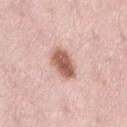Measured at roughly 4 mm in maximum diameter. Imaged with white-light lighting. Automated image analysis of the tile measured a footprint of about 8.5 mm², an outline eccentricity of about 0.75 (0 = round, 1 = elongated), and two-axis asymmetry of about 0.2. And it measured an average lesion color of about L≈59 a*≈23 b*≈28 (CIELAB), roughly 17 lightness units darker than nearby skin, and a normalized lesion–skin contrast near 10.5. The analysis additionally found a classifier nevus-likeness of about 100/100 and a lesion-detection confidence of about 100/100. A 15 mm close-up extracted from a 3D total-body photography capture. On the back. A female subject, about 50 years old.